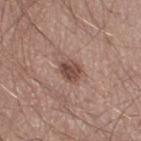  biopsy_status: not biopsied; imaged during a skin examination
  patient:
    sex: male
    age_approx: 30
  image:
    source: total-body photography crop
    field_of_view_mm: 15
  site: right thigh
  automated_metrics:
    border_irregularity_0_10: 2.0
    color_variation_0_10: 4.0
    peripheral_color_asymmetry: 1.5
  lighting: white-light